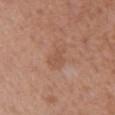The lesion was tiled from a total-body skin photograph and was not biopsied. Located on the abdomen. About 3.5 mm across. A close-up tile cropped from a whole-body skin photograph, about 15 mm across. The tile uses white-light illumination. A male subject roughly 65 years of age.